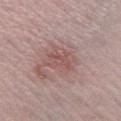Q: Is there a histopathology result?
A: imaged on a skin check; not biopsied
Q: Lesion size?
A: about 4 mm
Q: Lesion location?
A: the right lower leg
Q: Patient demographics?
A: male, aged approximately 40
Q: What kind of image is this?
A: 15 mm crop, total-body photography
Q: What lighting was used for the tile?
A: white-light